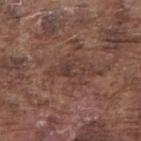biopsy status: imaged on a skin check; not biopsied | site: the right upper arm | TBP lesion metrics: a shape eccentricity near 0.75 and a shape-asymmetry score of about 0.45 (0 = symmetric); a normalized border contrast of about 5.5; a classifier nevus-likeness of about 0/100 | lighting: white-light | subject: male, aged 73–77 | image: 15 mm crop, total-body photography.